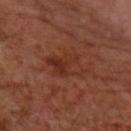Imaged during a routine full-body skin examination; the lesion was not biopsied and no histopathology is available. A female subject, in their mid- to late 60s. Measured at roughly 4.5 mm in maximum diameter. The lesion is on the right forearm. A 15 mm close-up extracted from a 3D total-body photography capture. Automated image analysis of the tile measured a footprint of about 10 mm², a shape eccentricity near 0.8, and two-axis asymmetry of about 0.3. And it measured a lesion–skin lightness drop of about 6. It also reported border irregularity of about 3.5 on a 0–10 scale and a color-variation rating of about 5.5/10. The tile uses cross-polarized illumination.